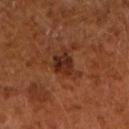Captured during whole-body skin photography for melanoma surveillance; the lesion was not biopsied. A close-up tile cropped from a whole-body skin photograph, about 15 mm across. A male subject about 65 years old. Approximately 4 mm at its widest.